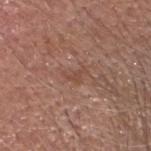Q: Is there a histopathology result?
A: imaged on a skin check; not biopsied
Q: What is the imaging modality?
A: total-body-photography crop, ~15 mm field of view
Q: Automated lesion metrics?
A: a lesion area of about 2.5 mm², an eccentricity of roughly 0.85, and a symmetry-axis asymmetry near 0.6; a mean CIELAB color near L≈47 a*≈21 b*≈27 and about 7 CIELAB-L* units darker than the surrounding skin; a border-irregularity rating of about 6.5/10, internal color variation of about 0 on a 0–10 scale, and radial color variation of about 0; lesion-presence confidence of about 90/100
Q: What are the patient's age and sex?
A: male, roughly 55 years of age
Q: Illumination type?
A: white-light illumination
Q: What is the lesion's diameter?
A: ≈3 mm
Q: Lesion location?
A: the head or neck